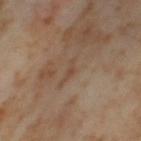Imaged during a routine full-body skin examination; the lesion was not biopsied and no histopathology is available.
A female patient, in their mid- to late 50s.
The lesion-visualizer software estimated a lesion color around L≈46 a*≈16 b*≈29 in CIELAB and a normalized border contrast of about 5.
Cropped from a total-body skin-imaging series; the visible field is about 15 mm.
Located on the left thigh.
Captured under cross-polarized illumination.
The lesion's longest dimension is about 2.5 mm.